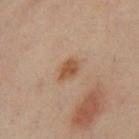Findings:
– biopsy status — no biopsy performed (imaged during a skin exam)
– automated lesion analysis — an average lesion color of about L≈53 a*≈21 b*≈34 (CIELAB), a lesion–skin lightness drop of about 10, and a normalized lesion–skin contrast near 8.5
– location — the left leg
– illumination — cross-polarized illumination
– image source — 15 mm crop, total-body photography
– subject — female, approximately 40 years of age
– size — about 3 mm